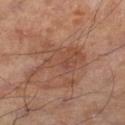follow-up: total-body-photography surveillance lesion; no biopsy
subject: male, in their mid-50s
acquisition: 15 mm crop, total-body photography
lesion diameter: ≈5.5 mm
body site: the leg